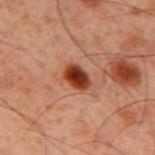The lesion was photographed on a routine skin check and not biopsied; there is no pathology result. The total-body-photography lesion software estimated a lesion color around L≈29 a*≈24 b*≈27 in CIELAB, about 15 CIELAB-L* units darker than the surrounding skin, and a lesion-to-skin contrast of about 13.5 (normalized; higher = more distinct). The software also gave an automated nevus-likeness rating near 100 out of 100 and a detector confidence of about 100 out of 100 that the crop contains a lesion. A lesion tile, about 15 mm wide, cut from a 3D total-body photograph. The lesion's longest dimension is about 3 mm. The lesion is on the mid back. This is a cross-polarized tile. A male patient, about 60 years old.